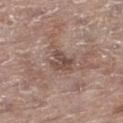Recorded during total-body skin imaging; not selected for excision or biopsy. The lesion is on the left lower leg. A female patient, about 85 years old or older. A 15 mm close-up tile from a total-body photography series done for melanoma screening. Imaged with white-light lighting. Measured at roughly 2.5 mm in maximum diameter.